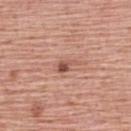Assessment:
This lesion was catalogued during total-body skin photography and was not selected for biopsy.
Image and clinical context:
The tile uses white-light illumination. Located on the upper back. A 15 mm close-up tile from a total-body photography series done for melanoma screening. A male patient aged 58–62.